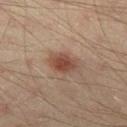Part of a total-body skin-imaging series; this lesion was reviewed on a skin check and was not flagged for biopsy. The subject is a male aged approximately 40. The total-body-photography lesion software estimated a mean CIELAB color near L≈41 a*≈18 b*≈24 and a normalized lesion–skin contrast near 8.5. The lesion's longest dimension is about 3.5 mm. This image is a 15 mm lesion crop taken from a total-body photograph. This is a cross-polarized tile. The lesion is on the left thigh.